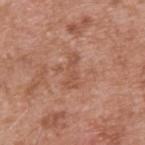Clinical impression: The lesion was photographed on a routine skin check and not biopsied; there is no pathology result. Acquisition and patient details: Automated tile analysis of the lesion measured a lesion color around L≈52 a*≈24 b*≈31 in CIELAB, a lesion–skin lightness drop of about 7, and a normalized border contrast of about 5. A male subject roughly 55 years of age. About 3.5 mm across. A lesion tile, about 15 mm wide, cut from a 3D total-body photograph. From the upper back. Imaged with white-light lighting.